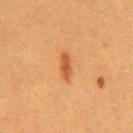Q: Was a biopsy performed?
A: no biopsy performed (imaged during a skin exam)
Q: How was this image acquired?
A: total-body-photography crop, ~15 mm field of view
Q: What did automated image analysis measure?
A: a border-irregularity index near 3.5/10, a color-variation rating of about 1/10, and peripheral color asymmetry of about 0.5
Q: Lesion location?
A: the chest
Q: How was the tile lit?
A: cross-polarized
Q: Patient demographics?
A: female, about 30 years old
Q: How large is the lesion?
A: about 3 mm